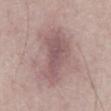The lesion was tiled from a total-body skin photograph and was not biopsied.
This is a white-light tile.
This image is a 15 mm lesion crop taken from a total-body photograph.
A male patient, in their 50s.
From the chest.